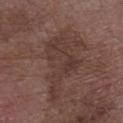Q: Is there a histopathology result?
A: catalogued during a skin exam; not biopsied
Q: Lesion size?
A: about 9 mm
Q: What is the imaging modality?
A: 15 mm crop, total-body photography
Q: Illumination type?
A: white-light
Q: Patient demographics?
A: male, aged 73 to 77
Q: What did automated image analysis measure?
A: a footprint of about 23 mm² and a shape eccentricity near 0.9; an average lesion color of about L≈36 a*≈18 b*≈21 (CIELAB), a lesion–skin lightness drop of about 6, and a normalized lesion–skin contrast near 5.5
Q: Where on the body is the lesion?
A: the arm